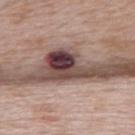biopsy status=total-body-photography surveillance lesion; no biopsy
patient=female, roughly 65 years of age
size=about 7 mm
site=the upper back
imaging modality=~15 mm crop, total-body skin-cancer survey
illumination=white-light illumination
TBP lesion metrics=an eccentricity of roughly 0.9 and a symmetry-axis asymmetry near 0.6; an average lesion color of about L≈41 a*≈19 b*≈18 (CIELAB), a lesion–skin lightness drop of about 21, and a normalized lesion–skin contrast near 15; a within-lesion color-variation index near 10/10 and peripheral color asymmetry of about 5